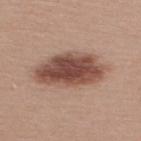<tbp_lesion>
<biopsy_status>not biopsied; imaged during a skin examination</biopsy_status>
<lesion_size>
  <long_diameter_mm_approx>8.0</long_diameter_mm_approx>
</lesion_size>
<lighting>white-light</lighting>
<image>
  <source>total-body photography crop</source>
  <field_of_view_mm>15</field_of_view_mm>
</image>
<patient>
  <sex>male</sex>
  <age_approx>40</age_approx>
</patient>
<site>upper back</site>
<automated_metrics>
  <eccentricity>0.85</eccentricity>
</automated_metrics>
</tbp_lesion>